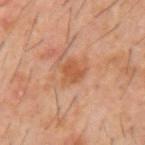Impression: The lesion was tiled from a total-body skin photograph and was not biopsied. Image and clinical context: The lesion is located on the mid back. The subject is a male about 60 years old. The total-body-photography lesion software estimated a lesion–skin lightness drop of about 8 and a lesion-to-skin contrast of about 7 (normalized; higher = more distinct). The software also gave a color-variation rating of about 1.5/10 and a peripheral color-asymmetry measure near 0.5. It also reported a classifier nevus-likeness of about 40/100 and a lesion-detection confidence of about 100/100. This is a cross-polarized tile. Measured at roughly 2.5 mm in maximum diameter. A 15 mm close-up extracted from a 3D total-body photography capture.